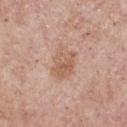<lesion>
  <biopsy_status>not biopsied; imaged during a skin examination</biopsy_status>
  <patient>
    <sex>male</sex>
    <age_approx>55</age_approx>
  </patient>
  <automated_metrics>
    <border_irregularity_0_10>2.5</border_irregularity_0_10>
    <color_variation_0_10>4.5</color_variation_0_10>
    <peripheral_color_asymmetry>1.5</peripheral_color_asymmetry>
  </automated_metrics>
  <image>
    <source>total-body photography crop</source>
    <field_of_view_mm>15</field_of_view_mm>
  </image>
  <site>chest</site>
</lesion>